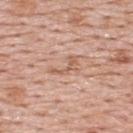| feature | finding |
|---|---|
| workup | catalogued during a skin exam; not biopsied |
| subject | male, aged around 55 |
| image-analysis metrics | a lesion color around L≈60 a*≈21 b*≈31 in CIELAB, about 8 CIELAB-L* units darker than the surrounding skin, and a normalized border contrast of about 5.5; a classifier nevus-likeness of about 0/100 and lesion-presence confidence of about 65/100 |
| tile lighting | white-light |
| diameter | ≈3.5 mm |
| site | the upper back |
| image | ~15 mm crop, total-body skin-cancer survey |